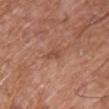{"image": {"source": "total-body photography crop", "field_of_view_mm": 15}, "patient": {"sex": "male", "age_approx": 60}, "site": "upper back"}